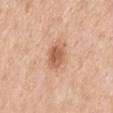biopsy status: imaged on a skin check; not biopsied | patient: female, aged around 40 | anatomic site: the back | size: about 4 mm | imaging modality: ~15 mm crop, total-body skin-cancer survey | TBP lesion metrics: an average lesion color of about L≈60 a*≈23 b*≈33 (CIELAB), roughly 12 lightness units darker than nearby skin, and a lesion-to-skin contrast of about 7.5 (normalized; higher = more distinct); border irregularity of about 2.5 on a 0–10 scale, a within-lesion color-variation index near 3.5/10, and radial color variation of about 1 | lighting: white-light illumination.